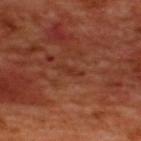Q: Is there a histopathology result?
A: catalogued during a skin exam; not biopsied
Q: What is the lesion's diameter?
A: about 3 mm
Q: Illumination type?
A: cross-polarized
Q: What kind of image is this?
A: 15 mm crop, total-body photography
Q: Patient demographics?
A: male, aged 48–52
Q: Where on the body is the lesion?
A: the upper back
Q: Automated lesion metrics?
A: an average lesion color of about L≈33 a*≈26 b*≈32 (CIELAB), roughly 5 lightness units darker than nearby skin, and a normalized border contrast of about 5.5; border irregularity of about 5 on a 0–10 scale, a within-lesion color-variation index near 0/10, and peripheral color asymmetry of about 0